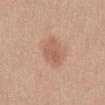Captured under white-light illumination. Approximately 3.5 mm at its widest. Located on the abdomen. A female patient, aged 28 to 32. Automated tile analysis of the lesion measured an outline eccentricity of about 0.75 (0 = round, 1 = elongated) and a shape-asymmetry score of about 0.35 (0 = symmetric). The analysis additionally found an automated nevus-likeness rating near 85 out of 100 and lesion-presence confidence of about 100/100. This image is a 15 mm lesion crop taken from a total-body photograph.